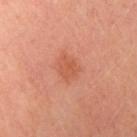workup: no biopsy performed (imaged during a skin exam) | anatomic site: the right upper arm | subject: female, about 35 years old | image: total-body-photography crop, ~15 mm field of view | automated metrics: a lesion color around L≈54 a*≈29 b*≈35 in CIELAB, a lesion–skin lightness drop of about 7, and a normalized border contrast of about 5.5; border irregularity of about 2.5 on a 0–10 scale and a within-lesion color-variation index near 1.5/10 | lesion diameter: about 2.5 mm.